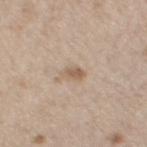<record>
<biopsy_status>not biopsied; imaged during a skin examination</biopsy_status>
<site>left thigh</site>
<automated_metrics>
  <area_mm2_approx>3.5</area_mm2_approx>
  <eccentricity>0.8</eccentricity>
  <shape_asymmetry>0.2</shape_asymmetry>
  <cielab_L>59</cielab_L>
  <cielab_a>15</cielab_a>
  <cielab_b>29</cielab_b>
  <vs_skin_darker_L>9.0</vs_skin_darker_L>
  <nevus_likeness_0_100>15</nevus_likeness_0_100>
  <lesion_detection_confidence_0_100>100</lesion_detection_confidence_0_100>
</automated_metrics>
<image>
  <source>total-body photography crop</source>
  <field_of_view_mm>15</field_of_view_mm>
</image>
<lighting>white-light</lighting>
<patient>
  <sex>male</sex>
  <age_approx>70</age_approx>
</patient>
</record>